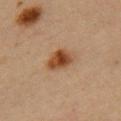biopsy_status: not biopsied; imaged during a skin examination
image:
  source: total-body photography crop
  field_of_view_mm: 15
patient:
  sex: female
  age_approx: 45
site: left upper arm